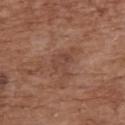follow-up = catalogued during a skin exam; not biopsied
subject = female, about 75 years old
automated lesion analysis = a lesion color around L≈44 a*≈21 b*≈27 in CIELAB, a lesion–skin lightness drop of about 6, and a normalized lesion–skin contrast near 5; a classifier nevus-likeness of about 0/100
anatomic site = the upper back
image = ~15 mm tile from a whole-body skin photo
lighting = white-light
lesion size = about 3.5 mm